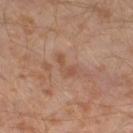Case summary:
• follow-up: no biopsy performed (imaged during a skin exam)
• body site: the left leg
• subject: male, aged around 30
• illumination: cross-polarized
• imaging modality: ~15 mm tile from a whole-body skin photo
• diameter: ~3 mm (longest diameter)